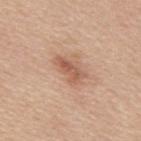Findings:
– biopsy status — catalogued during a skin exam; not biopsied